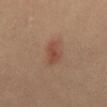This lesion was catalogued during total-body skin photography and was not selected for biopsy. Approximately 3.5 mm at its widest. A 15 mm close-up tile from a total-body photography series done for melanoma screening. Located on the abdomen. A male subject, in their 40s.